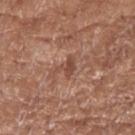Clinical summary:
A female subject, in their mid-70s. Located on the right forearm. A close-up tile cropped from a whole-body skin photograph, about 15 mm across. Captured under white-light illumination. Automated image analysis of the tile measured a nevus-likeness score of about 0/100 and a lesion-detection confidence of about 100/100.